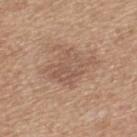Assessment:
The lesion was photographed on a routine skin check and not biopsied; there is no pathology result.
Acquisition and patient details:
A roughly 15 mm field-of-view crop from a total-body skin photograph. A male subject aged approximately 70. On the back.